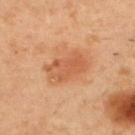acquisition: ~15 mm tile from a whole-body skin photo
size: ~5 mm (longest diameter)
automated metrics: a shape eccentricity near 0.8 and a shape-asymmetry score of about 0.2 (0 = symmetric); internal color variation of about 3.5 on a 0–10 scale
subject: male, aged around 55
anatomic site: the upper back
illumination: cross-polarized